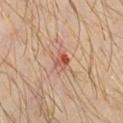A male subject, aged approximately 30.
The lesion's longest dimension is about 3 mm.
Automated image analysis of the tile measured a border-irregularity index near 3.5/10 and internal color variation of about 10 on a 0–10 scale. And it measured an automated nevus-likeness rating near 0 out of 100 and a lesion-detection confidence of about 100/100.
On the chest.
Cropped from a total-body skin-imaging series; the visible field is about 15 mm.